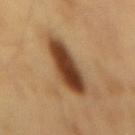Part of a total-body skin-imaging series; this lesion was reviewed on a skin check and was not flagged for biopsy. The subject is a male approximately 60 years of age. From the mid back. A region of skin cropped from a whole-body photographic capture, roughly 15 mm wide. The tile uses cross-polarized illumination. Approximately 7 mm at its widest.